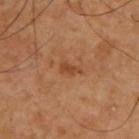Captured during whole-body skin photography for melanoma surveillance; the lesion was not biopsied. A male patient approximately 60 years of age. This is a cross-polarized tile. Measured at roughly 2.5 mm in maximum diameter. Automated tile analysis of the lesion measured a lesion area of about 2.5 mm², an outline eccentricity of about 0.85 (0 = round, 1 = elongated), and a symmetry-axis asymmetry near 0.35. The analysis additionally found a border-irregularity rating of about 3.5/10 and a within-lesion color-variation index near 0/10. The software also gave an automated nevus-likeness rating near 0 out of 100 and lesion-presence confidence of about 100/100. Located on the upper back. A region of skin cropped from a whole-body photographic capture, roughly 15 mm wide.